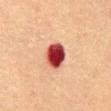Impression:
This lesion was catalogued during total-body skin photography and was not selected for biopsy.
Context:
The lesion is located on the front of the torso. Cropped from a total-body skin-imaging series; the visible field is about 15 mm. A female subject, about 55 years old. About 3.5 mm across. The tile uses cross-polarized illumination.